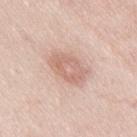Impression: Imaged during a routine full-body skin examination; the lesion was not biopsied and no histopathology is available. Context: Automated tile analysis of the lesion measured a footprint of about 9.5 mm², an eccentricity of roughly 0.8, and a shape-asymmetry score of about 0.2 (0 = symmetric). And it measured a border-irregularity rating of about 2.5/10, a color-variation rating of about 3/10, and radial color variation of about 1. The analysis additionally found a classifier nevus-likeness of about 5/100 and a lesion-detection confidence of about 100/100. A close-up tile cropped from a whole-body skin photograph, about 15 mm across. The tile uses white-light illumination. The lesion's longest dimension is about 4.5 mm. From the right thigh. A female subject, in their 40s.